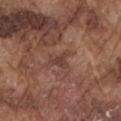Q: Was a biopsy performed?
A: total-body-photography surveillance lesion; no biopsy
Q: What is the anatomic site?
A: the left upper arm
Q: Illumination type?
A: white-light
Q: What kind of image is this?
A: ~15 mm crop, total-body skin-cancer survey
Q: Who is the patient?
A: male, aged 73 to 77
Q: How large is the lesion?
A: ≈2.5 mm